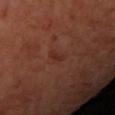The lesion was photographed on a routine skin check and not biopsied; there is no pathology result. The lesion is on the left forearm. This image is a 15 mm lesion crop taken from a total-body photograph. Imaged with cross-polarized lighting. Automated tile analysis of the lesion measured a border-irregularity index near 4.5/10 and a within-lesion color-variation index near 0/10. It also reported a detector confidence of about 100 out of 100 that the crop contains a lesion. A female subject, in their 50s.